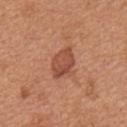Q: Is there a histopathology result?
A: no biopsy performed (imaged during a skin exam)
Q: Where on the body is the lesion?
A: the mid back
Q: Lesion size?
A: ≈4 mm
Q: What kind of image is this?
A: ~15 mm crop, total-body skin-cancer survey
Q: Patient demographics?
A: male, aged approximately 65
Q: What did automated image analysis measure?
A: a lesion area of about 8.5 mm² and an outline eccentricity of about 0.7 (0 = round, 1 = elongated); a border-irregularity index near 2/10 and internal color variation of about 3.5 on a 0–10 scale